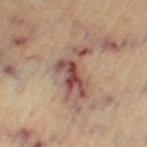Case summary:
- lesion diameter — ~6 mm (longest diameter)
- anatomic site — the left thigh
- patient — female, aged approximately 65
- imaging modality — ~15 mm tile from a whole-body skin photo
- illumination — cross-polarized illumination